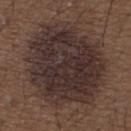Assessment:
The lesion was tiled from a total-body skin photograph and was not biopsied.
Context:
The lesion is located on the upper back. The lesion's longest dimension is about 10 mm. A region of skin cropped from a whole-body photographic capture, roughly 15 mm wide. A male patient in their 50s. The total-body-photography lesion software estimated a footprint of about 65 mm², an outline eccentricity of about 0.45 (0 = round, 1 = elongated), and a shape-asymmetry score of about 0.15 (0 = symmetric). And it measured border irregularity of about 2.5 on a 0–10 scale, internal color variation of about 4.5 on a 0–10 scale, and a peripheral color-asymmetry measure near 1.5.